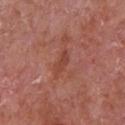Impression: No biopsy was performed on this lesion — it was imaged during a full skin examination and was not determined to be concerning. Background: The lesion is located on the chest. Approximately 3 mm at its widest. A close-up tile cropped from a whole-body skin photograph, about 15 mm across. The tile uses white-light illumination. A male patient aged approximately 65.